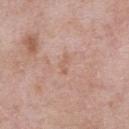notes = total-body-photography surveillance lesion; no biopsy
site = the chest
automated metrics = an automated nevus-likeness rating near 0 out of 100 and a lesion-detection confidence of about 100/100
acquisition = ~15 mm crop, total-body skin-cancer survey
lighting = white-light illumination
subject = male, aged approximately 55